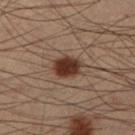Clinical impression:
The lesion was photographed on a routine skin check and not biopsied; there is no pathology result.
Acquisition and patient details:
The tile uses cross-polarized illumination. A 15 mm close-up tile from a total-body photography series done for melanoma screening. The lesion is on the leg. A male patient in their mid-50s.